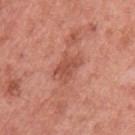Findings:
• biopsy status: total-body-photography surveillance lesion; no biopsy
• subject: female, about 50 years old
• body site: the arm
• TBP lesion metrics: an area of roughly 6.5 mm², an outline eccentricity of about 0.75 (0 = round, 1 = elongated), and a shape-asymmetry score of about 0.35 (0 = symmetric); a mean CIELAB color near L≈52 a*≈28 b*≈31, roughly 9 lightness units darker than nearby skin, and a normalized border contrast of about 6; an automated nevus-likeness rating near 0 out of 100 and a lesion-detection confidence of about 100/100
• lighting: white-light illumination
• lesion diameter: about 3.5 mm
• image: ~15 mm tile from a whole-body skin photo